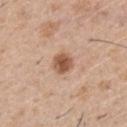| key | value |
|---|---|
| workup | imaged on a skin check; not biopsied |